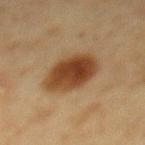{"biopsy_status": "not biopsied; imaged during a skin examination", "lighting": "cross-polarized", "automated_metrics": {"nevus_likeness_0_100": 100, "lesion_detection_confidence_0_100": 100}, "site": "mid back", "patient": {"sex": "female", "age_approx": 50}, "image": {"source": "total-body photography crop", "field_of_view_mm": 15}, "lesion_size": {"long_diameter_mm_approx": 6.0}}